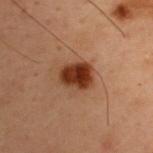Q: Was a biopsy performed?
A: imaged on a skin check; not biopsied